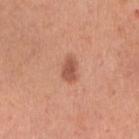Case summary:
– follow-up: imaged on a skin check; not biopsied
– lesion size: ~2.5 mm (longest diameter)
– tile lighting: white-light illumination
– location: the right upper arm
– patient: female, about 35 years old
– acquisition: total-body-photography crop, ~15 mm field of view